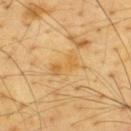biopsy status = no biopsy performed (imaged during a skin exam)
illumination = cross-polarized
body site = the upper back
acquisition = ~15 mm tile from a whole-body skin photo
diameter = ~3.5 mm (longest diameter)
TBP lesion metrics = a footprint of about 5 mm² and two-axis asymmetry of about 0.3; a lesion–skin lightness drop of about 6
subject = male, aged 63–67